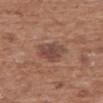| field | value |
|---|---|
| size | ≈4 mm |
| location | the upper back |
| image source | ~15 mm crop, total-body skin-cancer survey |
| lighting | white-light |
| subject | female, in their mid- to late 70s |